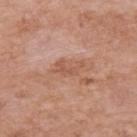{"biopsy_status": "not biopsied; imaged during a skin examination", "lesion_size": {"long_diameter_mm_approx": 3.5}, "image": {"source": "total-body photography crop", "field_of_view_mm": 15}, "lighting": "white-light", "patient": {"sex": "female", "age_approx": 70}, "site": "left upper arm"}